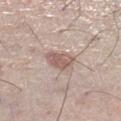No biopsy was performed on this lesion — it was imaged during a full skin examination and was not determined to be concerning. Measured at roughly 3.5 mm in maximum diameter. Cropped from a whole-body photographic skin survey; the tile spans about 15 mm. The lesion is located on the left lower leg. A male patient roughly 70 years of age.